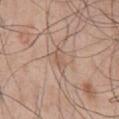No biopsy was performed on this lesion — it was imaged during a full skin examination and was not determined to be concerning.
The subject is a male aged 48–52.
Captured under white-light illumination.
The total-body-photography lesion software estimated a footprint of about 4 mm², an outline eccentricity of about 0.85 (0 = round, 1 = elongated), and two-axis asymmetry of about 0.3. It also reported a mean CIELAB color near L≈57 a*≈17 b*≈28 and a lesion-to-skin contrast of about 5 (normalized; higher = more distinct). It also reported a border-irregularity index near 3/10, a within-lesion color-variation index near 2/10, and peripheral color asymmetry of about 0.5. It also reported a classifier nevus-likeness of about 0/100 and lesion-presence confidence of about 65/100.
A roughly 15 mm field-of-view crop from a total-body skin photograph.
On the chest.
Approximately 3 mm at its widest.